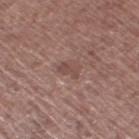Impression: The lesion was tiled from a total-body skin photograph and was not biopsied. Acquisition and patient details: Located on the right thigh. This is a white-light tile. Approximately 2.5 mm at its widest. The subject is a female aged around 50. This image is a 15 mm lesion crop taken from a total-body photograph.